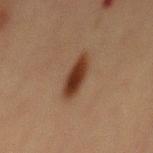Captured during whole-body skin photography for melanoma surveillance; the lesion was not biopsied. Cropped from a total-body skin-imaging series; the visible field is about 15 mm. The lesion-visualizer software estimated a border-irregularity index near 2.5/10 and radial color variation of about 1. And it measured a lesion-detection confidence of about 100/100. The patient is a male aged approximately 70. This is a cross-polarized tile. Measured at roughly 5 mm in maximum diameter. The lesion is on the front of the torso.